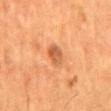The lesion was tiled from a total-body skin photograph and was not biopsied. The recorded lesion diameter is about 2.5 mm. The lesion is on the mid back. Cropped from a total-body skin-imaging series; the visible field is about 15 mm. A male patient, aged approximately 55. The total-body-photography lesion software estimated a footprint of about 4.5 mm² and a shape-asymmetry score of about 0.2 (0 = symmetric). The software also gave a lesion color around L≈54 a*≈26 b*≈39 in CIELAB, about 11 CIELAB-L* units darker than the surrounding skin, and a lesion-to-skin contrast of about 7 (normalized; higher = more distinct). The software also gave a border-irregularity index near 1.5/10, a color-variation rating of about 4/10, and peripheral color asymmetry of about 1.5. And it measured a classifier nevus-likeness of about 20/100 and lesion-presence confidence of about 100/100.